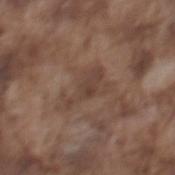| feature | finding |
|---|---|
| follow-up | no biopsy performed (imaged during a skin exam) |
| size | about 4.5 mm |
| subject | male, aged 73 to 77 |
| acquisition | 15 mm crop, total-body photography |
| body site | the mid back |
| tile lighting | white-light illumination |